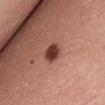{
  "biopsy_status": "not biopsied; imaged during a skin examination",
  "image": {
    "source": "total-body photography crop",
    "field_of_view_mm": 15
  },
  "lesion_size": {
    "long_diameter_mm_approx": 2.5
  },
  "patient": {
    "sex": "female",
    "age_approx": 35
  },
  "site": "abdomen"
}